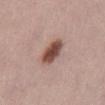Q: Was a biopsy performed?
A: no biopsy performed (imaged during a skin exam)